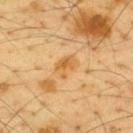workup = no biopsy performed (imaged during a skin exam) | size = about 3 mm | imaging modality = 15 mm crop, total-body photography | anatomic site = the upper back | automated lesion analysis = a nevus-likeness score of about 0/100 and a detector confidence of about 100 out of 100 that the crop contains a lesion | subject = male, approximately 65 years of age | tile lighting = cross-polarized illumination.